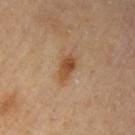Case summary:
• workup: catalogued during a skin exam; not biopsied
• lesion diameter: ≈3.5 mm
• lighting: cross-polarized illumination
• subject: male, about 85 years old or older
• body site: the left upper arm
• imaging modality: ~15 mm tile from a whole-body skin photo
• TBP lesion metrics: an area of roughly 5.5 mm² and a shape-asymmetry score of about 0.25 (0 = symmetric); a lesion color around L≈48 a*≈19 b*≈34 in CIELAB, roughly 10 lightness units darker than nearby skin, and a normalized border contrast of about 8; a lesion-detection confidence of about 100/100